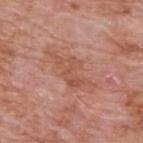workup: imaged on a skin check; not biopsied
body site: the upper back
lesion diameter: ≈6 mm
subject: male, aged around 60
image: ~15 mm tile from a whole-body skin photo
automated lesion analysis: an outline eccentricity of about 0.9 (0 = round, 1 = elongated)